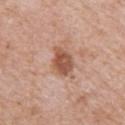Clinical summary: Located on the right upper arm. A lesion tile, about 15 mm wide, cut from a 3D total-body photograph. A female patient, approximately 70 years of age. Imaged with white-light lighting. The recorded lesion diameter is about 3.5 mm.